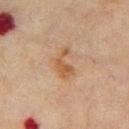Q: Was a biopsy performed?
A: no biopsy performed (imaged during a skin exam)
Q: Automated lesion metrics?
A: a footprint of about 6 mm² and a shape eccentricity near 0.85; a border-irregularity rating of about 6/10 and a color-variation rating of about 2/10
Q: Lesion location?
A: the chest
Q: Who is the patient?
A: male, approximately 70 years of age
Q: What is the imaging modality?
A: 15 mm crop, total-body photography
Q: How was the tile lit?
A: cross-polarized